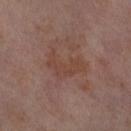• notes · catalogued during a skin exam; not biopsied
• image · total-body-photography crop, ~15 mm field of view
• site · the right thigh
• patient · female, approximately 55 years of age